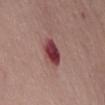| field | value |
|---|---|
| follow-up | catalogued during a skin exam; not biopsied |
| size | ≈3.5 mm |
| site | the mid back |
| lighting | white-light illumination |
| image-analysis metrics | a border-irregularity rating of about 2/10, internal color variation of about 5.5 on a 0–10 scale, and peripheral color asymmetry of about 2; an automated nevus-likeness rating near 0 out of 100 and a lesion-detection confidence of about 100/100 |
| patient | female, aged 68 to 72 |
| image | 15 mm crop, total-body photography |